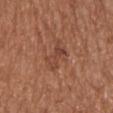No biopsy was performed on this lesion — it was imaged during a full skin examination and was not determined to be concerning. This is a white-light tile. A male patient in their 70s. Cropped from a total-body skin-imaging series; the visible field is about 15 mm. On the upper back. Longest diameter approximately 3.5 mm.